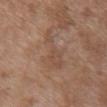| key | value |
|---|---|
| follow-up | catalogued during a skin exam; not biopsied |
| body site | the upper back |
| subject | female, aged around 70 |
| acquisition | 15 mm crop, total-body photography |
| lesion size | ≈6 mm |